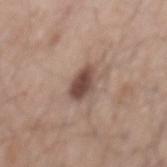Notes:
- body site · the mid back
- subject · male, roughly 55 years of age
- automated metrics · a nevus-likeness score of about 80/100 and a lesion-detection confidence of about 100/100
- image source · ~15 mm crop, total-body skin-cancer survey